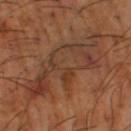Impression: Captured during whole-body skin photography for melanoma surveillance; the lesion was not biopsied. Acquisition and patient details: About 10.5 mm across. Captured under cross-polarized illumination. On the leg. A close-up tile cropped from a whole-body skin photograph, about 15 mm across. The patient is a male aged 63–67.